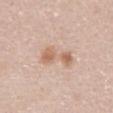This lesion was catalogued during total-body skin photography and was not selected for biopsy. The tile uses white-light illumination. The subject is a female in their 30s. An algorithmic analysis of the crop reported a mean CIELAB color near L≈63 a*≈19 b*≈30 and a lesion–skin lightness drop of about 10. A close-up tile cropped from a whole-body skin photograph, about 15 mm across. From the abdomen.